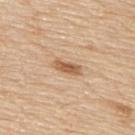Impression:
This lesion was catalogued during total-body skin photography and was not selected for biopsy.
Clinical summary:
The lesion is located on the upper back. Longest diameter approximately 3.5 mm. A male patient aged 78 to 82. Cropped from a total-body skin-imaging series; the visible field is about 15 mm. An algorithmic analysis of the crop reported an area of roughly 4.5 mm², an eccentricity of roughly 0.9, and a shape-asymmetry score of about 0.2 (0 = symmetric). It also reported a border-irregularity index near 2.5/10 and a color-variation rating of about 3/10. The software also gave a nevus-likeness score of about 85/100 and a lesion-detection confidence of about 100/100. Captured under white-light illumination.